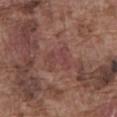Background: A 15 mm crop from a total-body photograph taken for skin-cancer surveillance. Longest diameter approximately 3 mm. Automated image analysis of the tile measured a mean CIELAB color near L≈42 a*≈23 b*≈21, a lesion–skin lightness drop of about 6, and a normalized lesion–skin contrast near 5. The analysis additionally found a border-irregularity index near 7.5/10, a color-variation rating of about 1/10, and a peripheral color-asymmetry measure near 0.5. And it measured a classifier nevus-likeness of about 0/100 and a lesion-detection confidence of about 90/100. The tile uses white-light illumination. A male subject, aged around 75. On the abdomen.